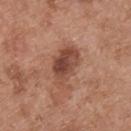| feature | finding |
|---|---|
| notes | catalogued during a skin exam; not biopsied |
| subject | male, roughly 55 years of age |
| image source | ~15 mm crop, total-body skin-cancer survey |
| site | the back |
| lesion size | ~5.5 mm (longest diameter) |
| lighting | white-light |